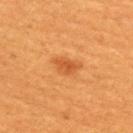Q: Was this lesion biopsied?
A: no biopsy performed (imaged during a skin exam)
Q: What is the anatomic site?
A: the upper back
Q: Automated lesion metrics?
A: a mean CIELAB color near L≈52 a*≈29 b*≈44, a lesion–skin lightness drop of about 9, and a normalized lesion–skin contrast near 6.5; border irregularity of about 3 on a 0–10 scale, internal color variation of about 2.5 on a 0–10 scale, and radial color variation of about 1; a classifier nevus-likeness of about 90/100 and a detector confidence of about 100 out of 100 that the crop contains a lesion
Q: Illumination type?
A: cross-polarized
Q: What is the imaging modality?
A: total-body-photography crop, ~15 mm field of view
Q: What are the patient's age and sex?
A: female, aged 28 to 32